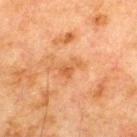Q: Was this lesion biopsied?
A: catalogued during a skin exam; not biopsied
Q: Lesion size?
A: ≈3 mm
Q: Patient demographics?
A: male, aged approximately 70
Q: What kind of image is this?
A: ~15 mm tile from a whole-body skin photo
Q: How was the tile lit?
A: cross-polarized illumination
Q: Where on the body is the lesion?
A: the upper back